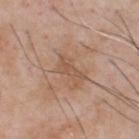Background:
The subject is a male roughly 50 years of age. The total-body-photography lesion software estimated a lesion color around L≈53 a*≈19 b*≈30 in CIELAB, about 7 CIELAB-L* units darker than the surrounding skin, and a lesion-to-skin contrast of about 5 (normalized; higher = more distinct). It also reported a color-variation rating of about 1/10. The software also gave a lesion-detection confidence of about 100/100. This is a white-light tile. A 15 mm crop from a total-body photograph taken for skin-cancer surveillance. From the front of the torso. Measured at roughly 3.5 mm in maximum diameter.